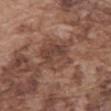Context:
A 15 mm close-up extracted from a 3D total-body photography capture. About 4.5 mm across. This is a white-light tile. The lesion is located on the abdomen. The patient is a male in their mid-70s.